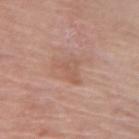The lesion is on the left upper arm. Measured at roughly 3.5 mm in maximum diameter. The total-body-photography lesion software estimated a mean CIELAB color near L≈57 a*≈21 b*≈28, roughly 6 lightness units darker than nearby skin, and a normalized lesion–skin contrast near 4.5. The software also gave border irregularity of about 5 on a 0–10 scale, a within-lesion color-variation index near 3/10, and radial color variation of about 1. The subject is a female approximately 65 years of age. A region of skin cropped from a whole-body photographic capture, roughly 15 mm wide. This is a white-light tile.